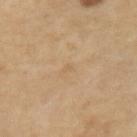site: the left arm | lesion diameter: about 1 mm | subject: female, aged approximately 40 | TBP lesion metrics: a lesion color around L≈60 a*≈15 b*≈35 in CIELAB and a normalized border contrast of about 3.5; border irregularity of about 3 on a 0–10 scale, a color-variation rating of about 0/10, and a peripheral color-asymmetry measure near 0 | lighting: cross-polarized illumination | image: 15 mm crop, total-body photography.